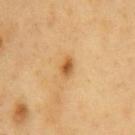The lesion is located on the chest. A roughly 15 mm field-of-view crop from a total-body skin photograph. A male subject roughly 60 years of age. The recorded lesion diameter is about 2.5 mm. The lesion-visualizer software estimated a nevus-likeness score of about 95/100.